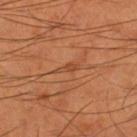workup: total-body-photography surveillance lesion; no biopsy | acquisition: 15 mm crop, total-body photography | anatomic site: the left thigh | image-analysis metrics: a footprint of about 2 mm², a shape eccentricity near 0.95, and a symmetry-axis asymmetry near 0.45; an average lesion color of about L≈45 a*≈25 b*≈36 (CIELAB), a lesion–skin lightness drop of about 7, and a normalized lesion–skin contrast near 5.5; a classifier nevus-likeness of about 0/100 and lesion-presence confidence of about 70/100 | size: about 2.5 mm | subject: male, aged 53 to 57.